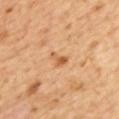Clinical summary:
Longest diameter approximately 2.5 mm. Captured under cross-polarized illumination. A 15 mm close-up extracted from a 3D total-body photography capture. A female patient roughly 55 years of age. The lesion is located on the back.